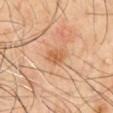Impression: The lesion was tiled from a total-body skin photograph and was not biopsied. Clinical summary: A male subject, in their mid-60s. A 15 mm close-up extracted from a 3D total-body photography capture. On the front of the torso.